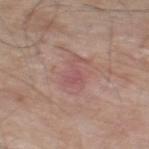Findings:
– notes — imaged on a skin check; not biopsied
– acquisition — total-body-photography crop, ~15 mm field of view
– subject — male, in their 80s
– anatomic site — the right thigh
– tile lighting — white-light illumination
– lesion size — about 3.5 mm